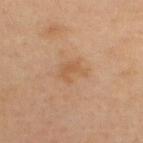Q: Was a biopsy performed?
A: imaged on a skin check; not biopsied
Q: Who is the patient?
A: male, aged 33 to 37
Q: Automated lesion metrics?
A: border irregularity of about 3.5 on a 0–10 scale, a within-lesion color-variation index near 2/10, and peripheral color asymmetry of about 1; a classifier nevus-likeness of about 0/100 and a lesion-detection confidence of about 100/100
Q: What is the anatomic site?
A: the upper back
Q: How was this image acquired?
A: total-body-photography crop, ~15 mm field of view